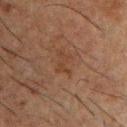Notes:
* notes: imaged on a skin check; not biopsied
* subject: male, aged 48–52
* illumination: cross-polarized
* image source: 15 mm crop, total-body photography
* size: ~3 mm (longest diameter)
* site: the front of the torso